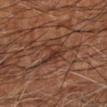Impression: Part of a total-body skin-imaging series; this lesion was reviewed on a skin check and was not flagged for biopsy. Image and clinical context: Located on the right forearm. A patient in their mid-60s. A 15 mm close-up extracted from a 3D total-body photography capture.